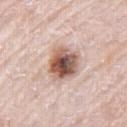workup — no biopsy performed (imaged during a skin exam); TBP lesion metrics — a nevus-likeness score of about 80/100; anatomic site — the right thigh; image source — 15 mm crop, total-body photography; tile lighting — white-light; subject — male, in their 80s; lesion diameter — ~4.5 mm (longest diameter).